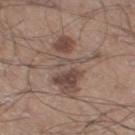The lesion is on the right thigh.
A roughly 15 mm field-of-view crop from a total-body skin photograph.
A male patient aged 48–52.
Automated image analysis of the tile measured a footprint of about 19 mm², an eccentricity of roughly 0.95, and a shape-asymmetry score of about 0.35 (0 = symmetric). And it measured a nevus-likeness score of about 10/100 and a detector confidence of about 100 out of 100 that the crop contains a lesion.
The lesion's longest dimension is about 8.5 mm.